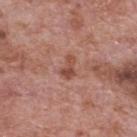• workup · total-body-photography surveillance lesion; no biopsy
• location · the mid back
• lesion size · about 3 mm
• illumination · white-light
• image source · ~15 mm tile from a whole-body skin photo
• patient · male, aged around 70
• automated lesion analysis · a lesion area of about 3.5 mm², a shape eccentricity near 0.85, and a symmetry-axis asymmetry near 0.45; a mean CIELAB color near L≈49 a*≈24 b*≈28 and a normalized lesion–skin contrast near 7; a border-irregularity index near 4/10, internal color variation of about 1.5 on a 0–10 scale, and radial color variation of about 0.5